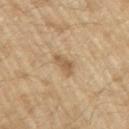| field | value |
|---|---|
| workup | total-body-photography surveillance lesion; no biopsy |
| illumination | white-light |
| imaging modality | ~15 mm crop, total-body skin-cancer survey |
| size | ~3 mm (longest diameter) |
| patient | male, aged around 70 |
| anatomic site | the right upper arm |
| automated lesion analysis | border irregularity of about 4 on a 0–10 scale and peripheral color asymmetry of about 1; a classifier nevus-likeness of about 30/100 and a lesion-detection confidence of about 100/100 |